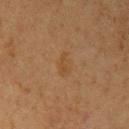notes: total-body-photography surveillance lesion; no biopsy
patient: female, in their 60s
tile lighting: cross-polarized
lesion diameter: ~3 mm (longest diameter)
TBP lesion metrics: a mean CIELAB color near L≈38 a*≈15 b*≈30, roughly 4 lightness units darker than nearby skin, and a lesion-to-skin contrast of about 4.5 (normalized; higher = more distinct); a border-irregularity rating of about 2.5/10, internal color variation of about 2 on a 0–10 scale, and radial color variation of about 0.5
site: the left upper arm
image: total-body-photography crop, ~15 mm field of view